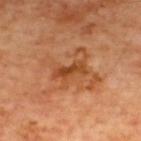- notes — no biopsy performed (imaged during a skin exam)
- anatomic site — the upper back
- lesion diameter — ≈4 mm
- image source — total-body-photography crop, ~15 mm field of view
- patient — male, aged around 60
- illumination — cross-polarized